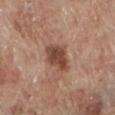The lesion is located on the left lower leg. The subject is a male aged around 70. The total-body-photography lesion software estimated a lesion area of about 9 mm², a shape eccentricity near 0.7, and two-axis asymmetry of about 0.2. The tile uses white-light illumination. A close-up tile cropped from a whole-body skin photograph, about 15 mm across.